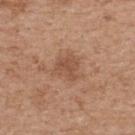Clinical impression: This lesion was catalogued during total-body skin photography and was not selected for biopsy. Background: A close-up tile cropped from a whole-body skin photograph, about 15 mm across. The patient is a female approximately 40 years of age. Longest diameter approximately 3 mm. On the upper back.